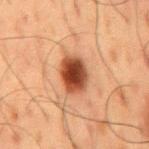Findings:
- biopsy status: total-body-photography surveillance lesion; no biopsy
- illumination: cross-polarized illumination
- image: 15 mm crop, total-body photography
- site: the mid back
- patient: male, aged 58–62
- lesion diameter: ~4 mm (longest diameter)